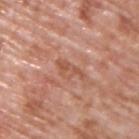| key | value |
|---|---|
| follow-up | total-body-photography surveillance lesion; no biopsy |
| automated metrics | a shape-asymmetry score of about 0.5 (0 = symmetric); a mean CIELAB color near L≈54 a*≈25 b*≈31 and a lesion-to-skin contrast of about 6 (normalized; higher = more distinct); a border-irregularity rating of about 6.5/10 and internal color variation of about 0.5 on a 0–10 scale |
| anatomic site | the upper back |
| subject | male, about 70 years old |
| lesion diameter | about 3.5 mm |
| image source | 15 mm crop, total-body photography |
| lighting | white-light |